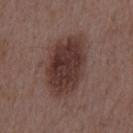This lesion was catalogued during total-body skin photography and was not selected for biopsy. A roughly 15 mm field-of-view crop from a total-body skin photograph. Located on the mid back. The subject is a male approximately 50 years of age.